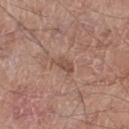biopsy status: imaged on a skin check; not biopsied | subject: male, in their 60s | diameter: ~3 mm (longest diameter) | illumination: white-light | body site: the right thigh | image: ~15 mm crop, total-body skin-cancer survey.